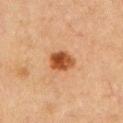Q: Where on the body is the lesion?
A: the right upper arm
Q: Who is the patient?
A: male, aged 63 to 67
Q: Lesion size?
A: ~3.5 mm (longest diameter)
Q: What is the imaging modality?
A: 15 mm crop, total-body photography